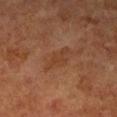The lesion is on the right upper arm. A roughly 15 mm field-of-view crop from a total-body skin photograph. A female patient, in their 60s.